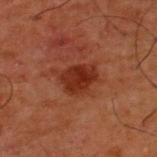workup: total-body-photography surveillance lesion; no biopsy | subject: male, in their 50s | site: the back | image: ~15 mm crop, total-body skin-cancer survey | illumination: cross-polarized.